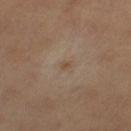- notes — imaged on a skin check; not biopsied
- patient — female
- acquisition — 15 mm crop, total-body photography
- automated metrics — a footprint of about 1 mm², a shape eccentricity near 0.75, and a shape-asymmetry score of about 0.4 (0 = symmetric); a lesion color around L≈48 a*≈16 b*≈29 in CIELAB, roughly 7 lightness units darker than nearby skin, and a normalized lesion–skin contrast near 6.5
- size — ~1 mm (longest diameter)